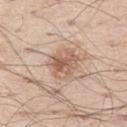{"image": {"source": "total-body photography crop", "field_of_view_mm": 15}, "patient": {"sex": "male", "age_approx": 60}, "site": "left thigh"}